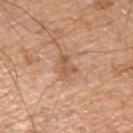The lesion was tiled from a total-body skin photograph and was not biopsied.
Approximately 2.5 mm at its widest.
A region of skin cropped from a whole-body photographic capture, roughly 15 mm wide.
A male patient, roughly 65 years of age.
Captured under white-light illumination.
The lesion is located on the arm.
The total-body-photography lesion software estimated a border-irregularity index near 5.5/10 and a color-variation rating of about 0/10. The analysis additionally found an automated nevus-likeness rating near 0 out of 100 and a lesion-detection confidence of about 100/100.